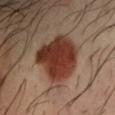A male subject, in their 40s. The lesion-visualizer software estimated a footprint of about 24 mm² and a symmetry-axis asymmetry near 0.2. And it measured a mean CIELAB color near L≈34 a*≈23 b*≈27 and a normalized lesion–skin contrast near 13. The software also gave an automated nevus-likeness rating near 100 out of 100 and a lesion-detection confidence of about 100/100. The recorded lesion diameter is about 6 mm. Cropped from a whole-body photographic skin survey; the tile spans about 15 mm. Captured under cross-polarized illumination. The lesion is located on the left forearm.